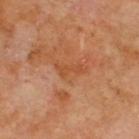Impression: Recorded during total-body skin imaging; not selected for excision or biopsy. Image and clinical context: This image is a 15 mm lesion crop taken from a total-body photograph. An algorithmic analysis of the crop reported an area of roughly 3.5 mm², a shape eccentricity near 0.9, and a symmetry-axis asymmetry near 0.4. And it measured an average lesion color of about L≈49 a*≈27 b*≈38 (CIELAB) and a lesion-to-skin contrast of about 5 (normalized; higher = more distinct). The subject is a male aged approximately 70. On the upper back. Imaged with cross-polarized lighting. Longest diameter approximately 3.5 mm.